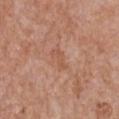workup — total-body-photography surveillance lesion; no biopsy | body site — the chest | patient — female, about 70 years old | image source — ~15 mm tile from a whole-body skin photo.